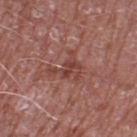follow-up: imaged on a skin check; not biopsied | image: 15 mm crop, total-body photography | patient: male, about 60 years old | site: the left thigh | lesion diameter: ~3.5 mm (longest diameter) | automated lesion analysis: a lesion area of about 6.5 mm² and a shape-asymmetry score of about 0.6 (0 = symmetric).